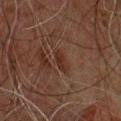Notes:
- follow-up · imaged on a skin check; not biopsied